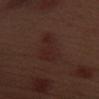workup: catalogued during a skin exam; not biopsied
image source: ~15 mm tile from a whole-body skin photo
TBP lesion metrics: a lesion area of about 11 mm², a shape eccentricity near 0.75, and a shape-asymmetry score of about 0.35 (0 = symmetric); a border-irregularity rating of about 4/10 and radial color variation of about 1
body site: the left upper arm
patient: male, approximately 70 years of age
lighting: white-light illumination
lesion size: ≈5 mm